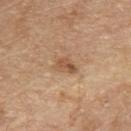follow-up = total-body-photography surveillance lesion; no biopsy | subject = male, aged 68–72 | anatomic site = the front of the torso | automated lesion analysis = a shape eccentricity near 0.75; a lesion color around L≈54 a*≈19 b*≈34 in CIELAB, roughly 10 lightness units darker than nearby skin, and a normalized border contrast of about 7; an automated nevus-likeness rating near 35 out of 100 and lesion-presence confidence of about 100/100 | diameter = ≈3 mm | illumination = white-light | imaging modality = ~15 mm crop, total-body skin-cancer survey.